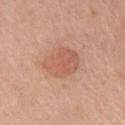Notes:
– biopsy status · total-body-photography surveillance lesion; no biopsy
– imaging modality · ~15 mm tile from a whole-body skin photo
– anatomic site · the left upper arm
– lesion diameter · ~4 mm (longest diameter)
– automated lesion analysis · a mean CIELAB color near L≈59 a*≈25 b*≈30, a lesion–skin lightness drop of about 8, and a normalized lesion–skin contrast near 5.5; border irregularity of about 1.5 on a 0–10 scale and a within-lesion color-variation index near 3/10
– tile lighting · white-light illumination
– subject · female, approximately 65 years of age